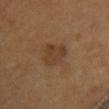Case summary:
- workup: catalogued during a skin exam; not biopsied
- lesion size: about 4 mm
- subject: female, roughly 65 years of age
- tile lighting: cross-polarized illumination
- location: the front of the torso
- automated lesion analysis: a footprint of about 7 mm², an outline eccentricity of about 0.7 (0 = round, 1 = elongated), and two-axis asymmetry of about 0.35; an average lesion color of about L≈35 a*≈16 b*≈29 (CIELAB) and a normalized border contrast of about 6; a nevus-likeness score of about 15/100
- image source: 15 mm crop, total-body photography